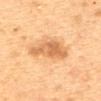Assessment: Captured during whole-body skin photography for melanoma surveillance; the lesion was not biopsied. Clinical summary: On the upper back. A roughly 15 mm field-of-view crop from a total-body skin photograph. A female subject, aged 48–52. Automated tile analysis of the lesion measured a lesion color around L≈55 a*≈20 b*≈36 in CIELAB, roughly 11 lightness units darker than nearby skin, and a normalized border contrast of about 7.5. And it measured border irregularity of about 4.5 on a 0–10 scale, a color-variation rating of about 3.5/10, and peripheral color asymmetry of about 1. Longest diameter approximately 5.5 mm. This is a cross-polarized tile.